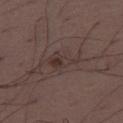Recorded during total-body skin imaging; not selected for excision or biopsy. On the leg. Automated tile analysis of the lesion measured an area of roughly 6 mm², an eccentricity of roughly 0.85, and two-axis asymmetry of about 0.5. The analysis additionally found about 6 CIELAB-L* units darker than the surrounding skin and a lesion-to-skin contrast of about 6.5 (normalized; higher = more distinct). It also reported a border-irregularity rating of about 6/10, internal color variation of about 4.5 on a 0–10 scale, and radial color variation of about 1.5. Cropped from a whole-body photographic skin survey; the tile spans about 15 mm. The patient is a male aged approximately 50. Imaged with white-light lighting.